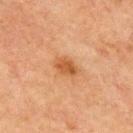workup=no biopsy performed (imaged during a skin exam) | lighting=cross-polarized | size=~3 mm (longest diameter) | location=the chest | imaging modality=~15 mm crop, total-body skin-cancer survey | subject=male, aged 63–67.